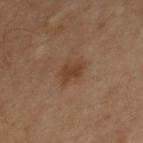Part of a total-body skin-imaging series; this lesion was reviewed on a skin check and was not flagged for biopsy.
A male patient, in their 30s.
A 15 mm close-up extracted from a 3D total-body photography capture.
Approximately 2.5 mm at its widest.
On the upper back.